The subject is a male in their mid- to late 60s, the lesion is on the head or neck, a 15 mm close-up extracted from a 3D total-body photography capture.
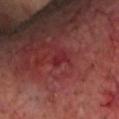The biopsy diagnosis was a skin cancer: nodular basal cell carcinoma.